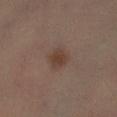<record>
  <biopsy_status>not biopsied; imaged during a skin examination</biopsy_status>
</record>